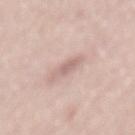Context: Measured at roughly 3.5 mm in maximum diameter. On the back. The lesion-visualizer software estimated a footprint of about 4 mm², an eccentricity of roughly 0.9, and a shape-asymmetry score of about 0.3 (0 = symmetric). The analysis additionally found a mean CIELAB color near L≈65 a*≈18 b*≈22 and about 9 CIELAB-L* units darker than the surrounding skin. And it measured a border-irregularity rating of about 3.5/10 and a color-variation rating of about 1/10. The analysis additionally found a nevus-likeness score of about 0/100 and a lesion-detection confidence of about 90/100. This image is a 15 mm lesion crop taken from a total-body photograph. The patient is a male about 60 years old. This is a white-light tile.